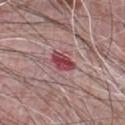{"image": {"source": "total-body photography crop", "field_of_view_mm": 15}, "lighting": "white-light", "site": "chest", "patient": {"sex": "male", "age_approx": 70}, "lesion_size": {"long_diameter_mm_approx": 3.0}}